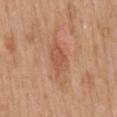Notes:
– notes — imaged on a skin check; not biopsied
– image-analysis metrics — a lesion area of about 6.5 mm² and a symmetry-axis asymmetry near 0.25; roughly 8 lightness units darker than nearby skin and a normalized lesion–skin contrast near 5.5; a nevus-likeness score of about 0/100 and a lesion-detection confidence of about 100/100
– image source — ~15 mm tile from a whole-body skin photo
– subject — female, aged approximately 40
– lesion diameter — ≈4 mm
– location — the mid back
– lighting — white-light illumination